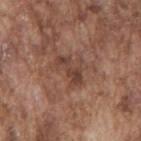Findings:
* follow-up — catalogued during a skin exam; not biopsied
* patient — male, aged 73–77
* imaging modality — 15 mm crop, total-body photography
* anatomic site — the mid back